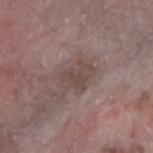Context:
The recorded lesion diameter is about 4 mm. Imaged with white-light lighting. A female patient, aged 63 to 67. A roughly 15 mm field-of-view crop from a total-body skin photograph. The lesion is on the right forearm. The total-body-photography lesion software estimated a border-irregularity rating of about 4/10 and radial color variation of about 1. The software also gave a nevus-likeness score of about 0/100.